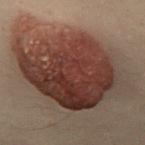<lesion>
  <biopsy_status>not biopsied; imaged during a skin examination</biopsy_status>
  <patient>
    <sex>male</sex>
    <age_approx>50</age_approx>
  </patient>
  <automated_metrics>
    <shape_asymmetry>0.2</shape_asymmetry>
    <cielab_L>29</cielab_L>
    <cielab_a>16</cielab_a>
    <cielab_b>20</cielab_b>
    <vs_skin_darker_L>12.0</vs_skin_darker_L>
    <vs_skin_contrast_norm>11.5</vs_skin_contrast_norm>
    <border_irregularity_0_10>3.5</border_irregularity_0_10>
    <color_variation_0_10>7.0</color_variation_0_10>
    <peripheral_color_asymmetry>2.0</peripheral_color_asymmetry>
  </automated_metrics>
  <lesion_size>
    <long_diameter_mm_approx>12.5</long_diameter_mm_approx>
  </lesion_size>
  <lighting>cross-polarized</lighting>
  <site>mid back</site>
  <image>
    <source>total-body photography crop</source>
    <field_of_view_mm>15</field_of_view_mm>
  </image>
</lesion>